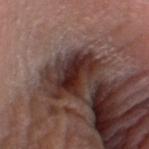No biopsy was performed on this lesion — it was imaged during a full skin examination and was not determined to be concerning. A male subject, aged 73 to 77. Automated image analysis of the tile measured a shape-asymmetry score of about 0.3 (0 = symmetric). It also reported a mean CIELAB color near L≈28 a*≈20 b*≈20 and a lesion–skin lightness drop of about 14. And it measured internal color variation of about 6.5 on a 0–10 scale and peripheral color asymmetry of about 2. Measured at roughly 6 mm in maximum diameter. Imaged with white-light lighting. The lesion is located on the head or neck. This image is a 15 mm lesion crop taken from a total-body photograph.